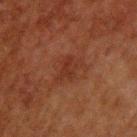Impression:
The lesion was tiled from a total-body skin photograph and was not biopsied.
Context:
Automated image analysis of the tile measured an outline eccentricity of about 0.7 (0 = round, 1 = elongated) and a shape-asymmetry score of about 0.25 (0 = symmetric). The software also gave roughly 5 lightness units darker than nearby skin and a normalized border contrast of about 5.5. The analysis additionally found a border-irregularity rating of about 2.5/10, a color-variation rating of about 1.5/10, and a peripheral color-asymmetry measure near 0.5. Captured under cross-polarized illumination. A roughly 15 mm field-of-view crop from a total-body skin photograph. The recorded lesion diameter is about 3 mm. The patient is a male aged approximately 60. From the upper back.